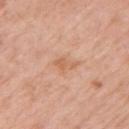Assessment:
Captured during whole-body skin photography for melanoma surveillance; the lesion was not biopsied.
Context:
The lesion-visualizer software estimated border irregularity of about 3 on a 0–10 scale, internal color variation of about 1.5 on a 0–10 scale, and a peripheral color-asymmetry measure near 0.5. The software also gave a lesion-detection confidence of about 100/100. The tile uses white-light illumination. The lesion is on the left upper arm. A female subject about 70 years old. Longest diameter approximately 2.5 mm. A 15 mm close-up extracted from a 3D total-body photography capture.